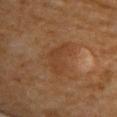<tbp_lesion>
<patient>
  <sex>female</sex>
  <age_approx>65</age_approx>
</patient>
<site>back</site>
<lesion_size>
  <long_diameter_mm_approx>5.0</long_diameter_mm_approx>
</lesion_size>
<image>
  <source>total-body photography crop</source>
  <field_of_view_mm>15</field_of_view_mm>
</image>
</tbp_lesion>